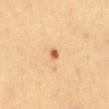workup = catalogued during a skin exam; not biopsied
patient = female, roughly 45 years of age
image-analysis metrics = a footprint of about 1.5 mm² and an eccentricity of roughly 0.55; an automated nevus-likeness rating near 95 out of 100 and a detector confidence of about 100 out of 100 that the crop contains a lesion
illumination = cross-polarized illumination
site = the abdomen
acquisition = total-body-photography crop, ~15 mm field of view